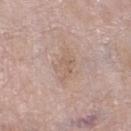follow-up — imaged on a skin check; not biopsied | image source — 15 mm crop, total-body photography | anatomic site — the right lower leg | automated metrics — an area of roughly 6.5 mm², an outline eccentricity of about 0.85 (0 = round, 1 = elongated), and two-axis asymmetry of about 0.35 | illumination — white-light illumination | patient — female, roughly 75 years of age.